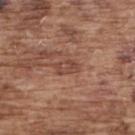Impression:
No biopsy was performed on this lesion — it was imaged during a full skin examination and was not determined to be concerning.